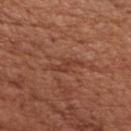biopsy status: no biopsy performed (imaged during a skin exam) | subject: male, in their mid- to late 60s | anatomic site: the chest | illumination: white-light | diameter: ≈4.5 mm | acquisition: ~15 mm tile from a whole-body skin photo.